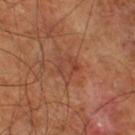Case summary:
– site — the leg
– patient — male, about 60 years old
– lesion diameter — ~3 mm (longest diameter)
– illumination — cross-polarized
– imaging modality — 15 mm crop, total-body photography